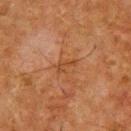Imaged during a routine full-body skin examination; the lesion was not biopsied and no histopathology is available.
Imaged with cross-polarized lighting.
A male patient, in their mid-60s.
A 15 mm close-up tile from a total-body photography series done for melanoma screening.
The lesion is located on the upper back.
The lesion's longest dimension is about 2.5 mm.
An algorithmic analysis of the crop reported a shape-asymmetry score of about 0.65 (0 = symmetric). The analysis additionally found a classifier nevus-likeness of about 0/100 and a lesion-detection confidence of about 100/100.